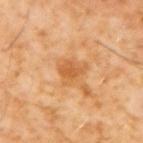biopsy_status: not biopsied; imaged during a skin examination
site: upper back
image:
  source: total-body photography crop
  field_of_view_mm: 15
patient:
  sex: male
  age_approx: 60
lighting: cross-polarized